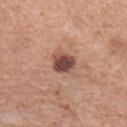automated metrics = an average lesion color of about L≈46 a*≈21 b*≈25 (CIELAB), roughly 16 lightness units darker than nearby skin, and a normalized border contrast of about 12
body site = the left forearm
image source = 15 mm crop, total-body photography
subject = female, in their 60s
size = ≈2.5 mm